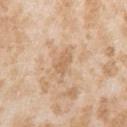The lesion was photographed on a routine skin check and not biopsied; there is no pathology result.
A 15 mm close-up tile from a total-body photography series done for melanoma screening.
The tile uses white-light illumination.
The total-body-photography lesion software estimated a footprint of about 3.5 mm² and an eccentricity of roughly 0.85. It also reported a lesion color around L≈63 a*≈18 b*≈35 in CIELAB, a lesion–skin lightness drop of about 7, and a normalized lesion–skin contrast near 5. The software also gave a color-variation rating of about 0.5/10 and radial color variation of about 0.
The lesion is located on the right upper arm.
A female subject approximately 25 years of age.
About 2.5 mm across.